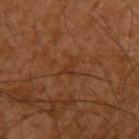Captured during whole-body skin photography for melanoma surveillance; the lesion was not biopsied.
The lesion-visualizer software estimated a lesion color around L≈33 a*≈22 b*≈31 in CIELAB, a lesion–skin lightness drop of about 5, and a lesion-to-skin contrast of about 5 (normalized; higher = more distinct). It also reported a classifier nevus-likeness of about 0/100 and lesion-presence confidence of about 95/100.
Longest diameter approximately 3 mm.
Cropped from a total-body skin-imaging series; the visible field is about 15 mm.
The lesion is on the upper back.
The subject is a male aged approximately 30.
The tile uses cross-polarized illumination.